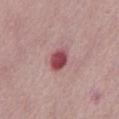Recorded during total-body skin imaging; not selected for excision or biopsy.
The patient is a male in their mid- to late 60s.
Located on the abdomen.
An algorithmic analysis of the crop reported a lesion area of about 5.5 mm². And it measured peripheral color asymmetry of about 1.5.
A 15 mm close-up tile from a total-body photography series done for melanoma screening.
The tile uses white-light illumination.
Longest diameter approximately 3 mm.